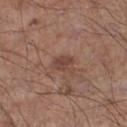The lesion was photographed on a routine skin check and not biopsied; there is no pathology result.
Automated image analysis of the tile measured a nevus-likeness score of about 0/100 and a detector confidence of about 100 out of 100 that the crop contains a lesion.
The lesion is located on the left lower leg.
A male subject, aged approximately 70.
This is a white-light tile.
The recorded lesion diameter is about 3 mm.
A roughly 15 mm field-of-view crop from a total-body skin photograph.